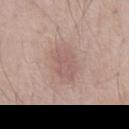| feature | finding |
|---|---|
| notes | catalogued during a skin exam; not biopsied |
| TBP lesion metrics | an area of roughly 9.5 mm² and an eccentricity of roughly 0.7; border irregularity of about 2 on a 0–10 scale, internal color variation of about 2.5 on a 0–10 scale, and radial color variation of about 1; a classifier nevus-likeness of about 0/100 and a detector confidence of about 100 out of 100 that the crop contains a lesion |
| lesion diameter | ≈4 mm |
| acquisition | 15 mm crop, total-body photography |
| patient | male, in their mid-50s |